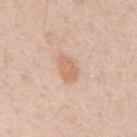Part of a total-body skin-imaging series; this lesion was reviewed on a skin check and was not flagged for biopsy. A lesion tile, about 15 mm wide, cut from a 3D total-body photograph. On the mid back. Measured at roughly 3.5 mm in maximum diameter. Captured under white-light illumination. A male subject, in their 50s. Automated image analysis of the tile measured a shape eccentricity near 0.75 and two-axis asymmetry of about 0.25. And it measured a border-irregularity index near 2.5/10 and a peripheral color-asymmetry measure near 1.